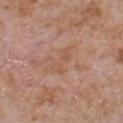Notes:
* biopsy status — total-body-photography surveillance lesion; no biopsy
* lighting — white-light illumination
* site — the front of the torso
* patient — male, roughly 65 years of age
* image-analysis metrics — an average lesion color of about L≈55 a*≈21 b*≈31 (CIELAB) and about 5 CIELAB-L* units darker than the surrounding skin; a border-irregularity index near 4.5/10, internal color variation of about 2 on a 0–10 scale, and a peripheral color-asymmetry measure near 0.5
* image — total-body-photography crop, ~15 mm field of view